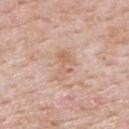{"biopsy_status": "not biopsied; imaged during a skin examination", "site": "upper back", "image": {"source": "total-body photography crop", "field_of_view_mm": 15}, "patient": {"sex": "male", "age_approx": 50}, "lesion_size": {"long_diameter_mm_approx": 4.0}, "lighting": "white-light"}